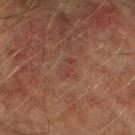{
  "biopsy_status": "not biopsied; imaged during a skin examination",
  "lesion_size": {
    "long_diameter_mm_approx": 2.5
  },
  "image": {
    "source": "total-body photography crop",
    "field_of_view_mm": 15
  },
  "patient": {
    "sex": "male",
    "age_approx": 75
  },
  "automated_metrics": {
    "cielab_L": 32,
    "cielab_a": 19,
    "cielab_b": 22,
    "vs_skin_darker_L": 4.0,
    "vs_skin_contrast_norm": 5.0,
    "color_variation_0_10": 0.0,
    "peripheral_color_asymmetry": 0.0
  },
  "site": "leg"
}